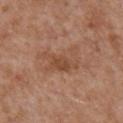Assessment:
The lesion was tiled from a total-body skin photograph and was not biopsied.
Clinical summary:
An algorithmic analysis of the crop reported a footprint of about 10 mm² and a shape-asymmetry score of about 0.4 (0 = symmetric). The software also gave a mean CIELAB color near L≈49 a*≈21 b*≈31, about 7 CIELAB-L* units darker than the surrounding skin, and a normalized border contrast of about 6. Imaged with white-light lighting. A 15 mm crop from a total-body photograph taken for skin-cancer surveillance. A male patient approximately 65 years of age. The lesion is located on the chest.